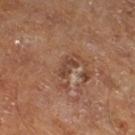The lesion was tiled from a total-body skin photograph and was not biopsied. A lesion tile, about 15 mm wide, cut from a 3D total-body photograph. The lesion-visualizer software estimated a shape eccentricity near 0.85 and a symmetry-axis asymmetry near 0.4. The analysis additionally found roughly 8 lightness units darker than nearby skin and a normalized border contrast of about 6.5. The software also gave a border-irregularity rating of about 4.5/10 and radial color variation of about 0. This is a cross-polarized tile. The lesion is located on the right thigh. A male subject, aged 63–67. Approximately 2.5 mm at its widest.